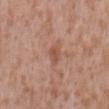<case>
<biopsy_status>not biopsied; imaged during a skin examination</biopsy_status>
<site>back</site>
<lighting>white-light</lighting>
<automated_metrics>
  <cielab_L>52</cielab_L>
  <cielab_a>22</cielab_a>
  <cielab_b>29</cielab_b>
  <vs_skin_darker_L>8.0</vs_skin_darker_L>
  <vs_skin_contrast_norm>6.0</vs_skin_contrast_norm>
  <border_irregularity_0_10>2.0</border_irregularity_0_10>
  <color_variation_0_10>1.5</color_variation_0_10>
  <peripheral_color_asymmetry>0.5</peripheral_color_asymmetry>
</automated_metrics>
<lesion_size>
  <long_diameter_mm_approx>2.5</long_diameter_mm_approx>
</lesion_size>
<image>
  <source>total-body photography crop</source>
  <field_of_view_mm>15</field_of_view_mm>
</image>
<patient>
  <sex>male</sex>
  <age_approx>45</age_approx>
</patient>
</case>